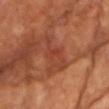<tbp_lesion>
  <biopsy_status>not biopsied; imaged during a skin examination</biopsy_status>
  <image>
    <source>total-body photography crop</source>
    <field_of_view_mm>15</field_of_view_mm>
  </image>
  <patient>
    <sex>male</sex>
    <age_approx>60</age_approx>
  </patient>
  <site>chest</site>
</tbp_lesion>